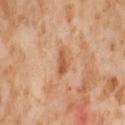Findings:
• workup: total-body-photography surveillance lesion; no biopsy
• image-analysis metrics: a lesion area of about 4.5 mm²; a lesion–skin lightness drop of about 10 and a normalized border contrast of about 7; border irregularity of about 3 on a 0–10 scale, a color-variation rating of about 3/10, and radial color variation of about 1
• diameter: ≈3 mm
• illumination: cross-polarized
• anatomic site: the abdomen
• subject: female, about 55 years old
• image: 15 mm crop, total-body photography